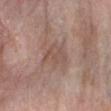No biopsy was performed on this lesion — it was imaged during a full skin examination and was not determined to be concerning. A region of skin cropped from a whole-body photographic capture, roughly 15 mm wide. A female patient about 65 years old. Captured under white-light illumination. Located on the arm. The total-body-photography lesion software estimated an average lesion color of about L≈51 a*≈18 b*≈24 (CIELAB), a lesion–skin lightness drop of about 6, and a normalized lesion–skin contrast near 5. And it measured a classifier nevus-likeness of about 0/100 and lesion-presence confidence of about 95/100.